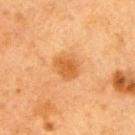Assessment:
This lesion was catalogued during total-body skin photography and was not selected for biopsy.
Acquisition and patient details:
The patient is a female in their 40s. Imaged with cross-polarized lighting. A 15 mm crop from a total-body photograph taken for skin-cancer surveillance. Longest diameter approximately 3 mm. The lesion is located on the right upper arm.